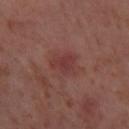Case summary:
* workup — catalogued during a skin exam; not biopsied
* automated lesion analysis — a classifier nevus-likeness of about 0/100 and a detector confidence of about 100 out of 100 that the crop contains a lesion
* subject — female, aged around 55
* image — ~15 mm crop, total-body skin-cancer survey
* illumination — cross-polarized illumination
* site — the right thigh
* diameter — about 3 mm